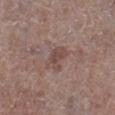<case>
  <biopsy_status>not biopsied; imaged during a skin examination</biopsy_status>
</case>